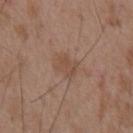Assessment: The lesion was tiled from a total-body skin photograph and was not biopsied. Image and clinical context: Measured at roughly 3 mm in maximum diameter. From the abdomen. A 15 mm close-up extracted from a 3D total-body photography capture. A male patient, about 55 years old. The tile uses white-light illumination.